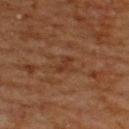{
  "biopsy_status": "not biopsied; imaged during a skin examination",
  "site": "upper back",
  "lesion_size": {
    "long_diameter_mm_approx": 2.5
  },
  "image": {
    "source": "total-body photography crop",
    "field_of_view_mm": 15
  },
  "patient": {
    "sex": "male",
    "age_approx": 65
  },
  "lighting": "cross-polarized"
}